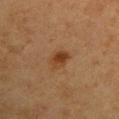image source: total-body-photography crop, ~15 mm field of view
anatomic site: the left upper arm
tile lighting: cross-polarized
image-analysis metrics: a shape eccentricity near 0.8 and two-axis asymmetry of about 0.25; an automated nevus-likeness rating near 95 out of 100 and a detector confidence of about 100 out of 100 that the crop contains a lesion
lesion size: ≈3.5 mm
patient: female, approximately 40 years of age